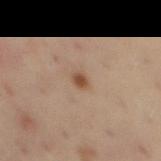The lesion was photographed on a routine skin check and not biopsied; there is no pathology result. The tile uses cross-polarized illumination. This image is a 15 mm lesion crop taken from a total-body photograph. The subject is a male aged around 45. The lesion's longest dimension is about 2 mm. On the mid back.